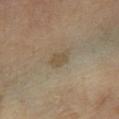Findings:
• patient: male, roughly 65 years of age
• lighting: cross-polarized
• anatomic site: the left lower leg
• image source: ~15 mm tile from a whole-body skin photo
• automated lesion analysis: a shape eccentricity near 0.75 and a symmetry-axis asymmetry near 0.3; a mean CIELAB color near L≈48 a*≈9 b*≈28, about 7 CIELAB-L* units darker than the surrounding skin, and a normalized border contrast of about 6; a border-irregularity index near 3/10, internal color variation of about 1.5 on a 0–10 scale, and peripheral color asymmetry of about 0.5
• lesion diameter: ≈2.5 mm